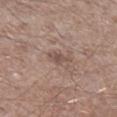| field | value |
|---|---|
| workup | no biopsy performed (imaged during a skin exam) |
| image source | ~15 mm crop, total-body skin-cancer survey |
| site | the leg |
| TBP lesion metrics | a footprint of about 3 mm², an outline eccentricity of about 0.85 (0 = round, 1 = elongated), and two-axis asymmetry of about 0.25; a border-irregularity rating of about 3/10 and internal color variation of about 1.5 on a 0–10 scale |
| patient | male, aged 63–67 |
| lesion diameter | about 3 mm |